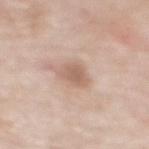acquisition — ~15 mm tile from a whole-body skin photo
subject — female, aged approximately 50
site — the upper back
tile lighting — white-light illumination
size — ~3 mm (longest diameter)